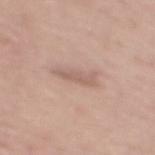Background:
Measured at roughly 4 mm in maximum diameter. A 15 mm close-up tile from a total-body photography series done for melanoma screening. From the mid back. Automated image analysis of the tile measured an eccentricity of roughly 0.95 and a shape-asymmetry score of about 0.3 (0 = symmetric). And it measured border irregularity of about 4.5 on a 0–10 scale, a color-variation rating of about 0/10, and peripheral color asymmetry of about 0. It also reported a classifier nevus-likeness of about 0/100 and lesion-presence confidence of about 95/100. A female subject, in their mid- to late 60s. Captured under white-light illumination.